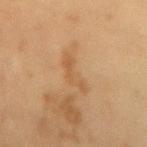The lesion is located on the lower back.
Measured at roughly 4.5 mm in maximum diameter.
This image is a 15 mm lesion crop taken from a total-body photograph.
A male subject, aged 58–62.